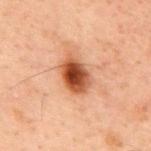This image is a 15 mm lesion crop taken from a total-body photograph. Approximately 4 mm at its widest. A male patient, aged around 60. Captured under cross-polarized illumination. From the upper back.